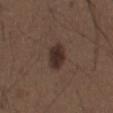workup=no biopsy performed (imaged during a skin exam) | diameter=about 4 mm | body site=the abdomen | patient=male, in their 50s | acquisition=total-body-photography crop, ~15 mm field of view.